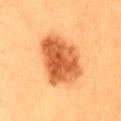| feature | finding |
|---|---|
| biopsy status | total-body-photography surveillance lesion; no biopsy |
| image source | total-body-photography crop, ~15 mm field of view |
| location | the lower back |
| subject | female, approximately 40 years of age |
| lighting | cross-polarized |
| diameter | ≈6.5 mm |